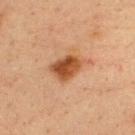Recorded during total-body skin imaging; not selected for excision or biopsy. Cropped from a total-body skin-imaging series; the visible field is about 15 mm. The lesion is located on the back. A male patient roughly 35 years of age.